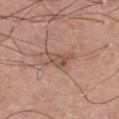workup: catalogued during a skin exam; not biopsied | image source: total-body-photography crop, ~15 mm field of view | location: the right thigh | lesion size: ≈3 mm | image-analysis metrics: a nevus-likeness score of about 0/100 | subject: male, aged approximately 75 | tile lighting: white-light illumination.